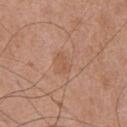A region of skin cropped from a whole-body photographic capture, roughly 15 mm wide. A male subject, aged 73 to 77. The lesion is located on the chest.